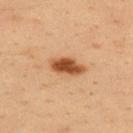Case summary:
- notes: imaged on a skin check; not biopsied
- lesion size: ≈4.5 mm
- body site: the upper back
- illumination: cross-polarized
- patient: male, aged 33–37
- acquisition: total-body-photography crop, ~15 mm field of view